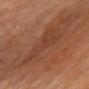Assessment: Recorded during total-body skin imaging; not selected for excision or biopsy. Background: Approximately 6.5 mm at its widest. The lesion-visualizer software estimated an area of roughly 12 mm², an outline eccentricity of about 0.9 (0 = round, 1 = elongated), and a shape-asymmetry score of about 0.55 (0 = symmetric). And it measured an average lesion color of about L≈34 a*≈20 b*≈27 (CIELAB) and a lesion-to-skin contrast of about 4.5 (normalized; higher = more distinct). It also reported border irregularity of about 7 on a 0–10 scale, a within-lesion color-variation index near 2/10, and radial color variation of about 0.5. A 15 mm close-up tile from a total-body photography series done for melanoma screening. The tile uses cross-polarized illumination. The subject is a female approximately 80 years of age. The lesion is located on the chest.